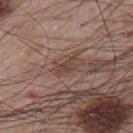Case summary:
* notes — imaged on a skin check; not biopsied
* illumination — white-light
* site — the left upper arm
* automated lesion analysis — a lesion area of about 3.5 mm², a shape eccentricity near 0.8, and two-axis asymmetry of about 0.45; a lesion color around L≈43 a*≈18 b*≈25 in CIELAB and a lesion–skin lightness drop of about 8
* lesion diameter — ≈2.5 mm
* imaging modality — total-body-photography crop, ~15 mm field of view
* patient — male, approximately 65 years of age